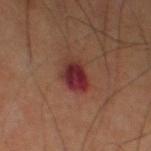The lesion was photographed on a routine skin check and not biopsied; there is no pathology result.
The lesion is located on the right thigh.
About 3.5 mm across.
A roughly 15 mm field-of-view crop from a total-body skin photograph.
A male subject aged approximately 60.